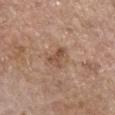This lesion was catalogued during total-body skin photography and was not selected for biopsy. Imaged with white-light lighting. Located on the left forearm. A close-up tile cropped from a whole-body skin photograph, about 15 mm across. The lesion's longest dimension is about 3.5 mm. A female subject in their mid- to late 60s.